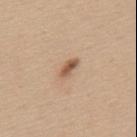Part of a total-body skin-imaging series; this lesion was reviewed on a skin check and was not flagged for biopsy. Cropped from a total-body skin-imaging series; the visible field is about 15 mm. A female patient, roughly 40 years of age. The lesion is located on the mid back.